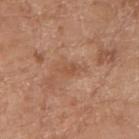The subject is a female aged around 75.
Imaged with white-light lighting.
The total-body-photography lesion software estimated about 7 CIELAB-L* units darker than the surrounding skin and a lesion-to-skin contrast of about 5 (normalized; higher = more distinct). It also reported a border-irregularity rating of about 3.5/10 and a within-lesion color-variation index near 1/10. The analysis additionally found a lesion-detection confidence of about 100/100.
The lesion's longest dimension is about 3 mm.
The lesion is on the left forearm.
A close-up tile cropped from a whole-body skin photograph, about 15 mm across.